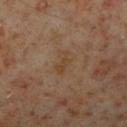– follow-up — total-body-photography surveillance lesion; no biopsy
– patient — male, approximately 60 years of age
– image — total-body-photography crop, ~15 mm field of view
– body site — the right lower leg